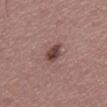| key | value |
|---|---|
| biopsy status | total-body-photography surveillance lesion; no biopsy |
| lesion diameter | ≈3 mm |
| automated metrics | a lesion area of about 4.5 mm², an outline eccentricity of about 0.8 (0 = round, 1 = elongated), and a shape-asymmetry score of about 0.2 (0 = symmetric); a border-irregularity index near 2.5/10 and radial color variation of about 1.5; a classifier nevus-likeness of about 85/100 |
| subject | male, about 40 years old |
| acquisition | 15 mm crop, total-body photography |
| lighting | white-light illumination |
| body site | the abdomen |